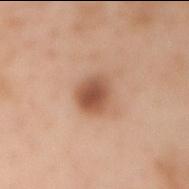Impression: The lesion was photographed on a routine skin check and not biopsied; there is no pathology result.